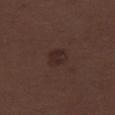notes = no biopsy performed (imaged during a skin exam) | anatomic site = the right thigh | subject = female, aged approximately 50 | imaging modality = ~15 mm tile from a whole-body skin photo | lighting = white-light.